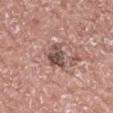biopsy_status: not biopsied; imaged during a skin examination
automated_metrics:
  area_mm2_approx: 6.5
  eccentricity: 0.45
  shape_asymmetry: 0.35
  vs_skin_darker_L: 13.0
  border_irregularity_0_10: 3.5
  color_variation_0_10: 6.0
  peripheral_color_asymmetry: 2.0
  nevus_likeness_0_100: 0
  lesion_detection_confidence_0_100: 100
image:
  source: total-body photography crop
  field_of_view_mm: 15
site: back
lesion_size:
  long_diameter_mm_approx: 3.0
lighting: white-light
patient:
  sex: male
  age_approx: 70